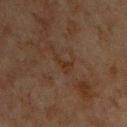The lesion was photographed on a routine skin check and not biopsied; there is no pathology result. The recorded lesion diameter is about 2.5 mm. A 15 mm crop from a total-body photograph taken for skin-cancer surveillance. The lesion is on the upper back. A male subject about 65 years old.